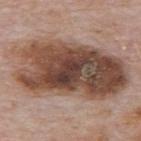Imaged during a routine full-body skin examination; the lesion was not biopsied and no histopathology is available.
The subject is a male aged 73 to 77.
The lesion is on the mid back.
The lesion-visualizer software estimated a footprint of about 65 mm², an outline eccentricity of about 0.85 (0 = round, 1 = elongated), and a symmetry-axis asymmetry near 0.15. And it measured a border-irregularity index near 2.5/10, a color-variation rating of about 9.5/10, and a peripheral color-asymmetry measure near 3.5. The analysis additionally found an automated nevus-likeness rating near 55 out of 100 and a detector confidence of about 100 out of 100 that the crop contains a lesion.
Measured at roughly 13 mm in maximum diameter.
This image is a 15 mm lesion crop taken from a total-body photograph.
Imaged with white-light lighting.